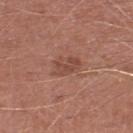Recorded during total-body skin imaging; not selected for excision or biopsy. The tile uses white-light illumination. The subject is a male about 65 years old. On the left lower leg. Approximately 3.5 mm at its widest. A 15 mm close-up tile from a total-body photography series done for melanoma screening. The total-body-photography lesion software estimated an area of roughly 6 mm², an eccentricity of roughly 0.8, and two-axis asymmetry of about 0.25. The analysis additionally found a detector confidence of about 100 out of 100 that the crop contains a lesion.